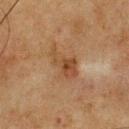  biopsy_status: not biopsied; imaged during a skin examination
  image:
    source: total-body photography crop
    field_of_view_mm: 15
  patient:
    sex: male
    age_approx: 75
  site: chest